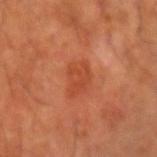image:
  source: total-body photography crop
  field_of_view_mm: 15
site: right forearm
lighting: cross-polarized
patient:
  sex: male
  age_approx: 60
lesion_size:
  long_diameter_mm_approx: 3.5